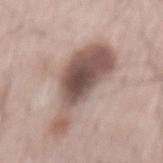Q: How large is the lesion?
A: ~10 mm (longest diameter)
Q: What kind of image is this?
A: ~15 mm crop, total-body skin-cancer survey
Q: Lesion location?
A: the back
Q: What are the patient's age and sex?
A: male, in their mid- to late 60s
Q: Automated lesion metrics?
A: an eccentricity of roughly 0.95 and a shape-asymmetry score of about 0.5 (0 = symmetric); a border-irregularity rating of about 6/10 and a color-variation rating of about 6/10
Q: What lighting was used for the tile?
A: white-light illumination